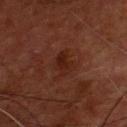Q: Was a biopsy performed?
A: catalogued during a skin exam; not biopsied
Q: What are the patient's age and sex?
A: male, approximately 55 years of age
Q: What is the imaging modality?
A: ~15 mm crop, total-body skin-cancer survey
Q: What did automated image analysis measure?
A: a lesion color around L≈21 a*≈22 b*≈24 in CIELAB and roughly 6 lightness units darker than nearby skin; a classifier nevus-likeness of about 0/100
Q: How was the tile lit?
A: cross-polarized illumination
Q: Lesion location?
A: the chest
Q: How large is the lesion?
A: ~2.5 mm (longest diameter)